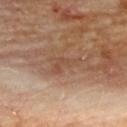Imaged during a routine full-body skin examination; the lesion was not biopsied and no histopathology is available.
A lesion tile, about 15 mm wide, cut from a 3D total-body photograph.
A male subject aged 83 or older.
Approximately 3.5 mm at its widest.
The lesion-visualizer software estimated a mean CIELAB color near L≈47 a*≈19 b*≈29 and a lesion-to-skin contrast of about 5 (normalized; higher = more distinct). The software also gave a border-irregularity index near 7/10, a color-variation rating of about 1/10, and a peripheral color-asymmetry measure near 0.
On the upper back.